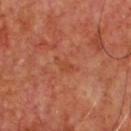The lesion was photographed on a routine skin check and not biopsied; there is no pathology result.
From the chest.
The subject is a male in their mid-60s.
Approximately 3 mm at its widest.
This is a cross-polarized tile.
A roughly 15 mm field-of-view crop from a total-body skin photograph.